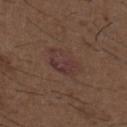Part of a total-body skin-imaging series; this lesion was reviewed on a skin check and was not flagged for biopsy. An algorithmic analysis of the crop reported a lesion color around L≈34 a*≈18 b*≈20 in CIELAB, roughly 5 lightness units darker than nearby skin, and a normalized border contrast of about 6. The tile uses white-light illumination. Measured at roughly 4 mm in maximum diameter. A male patient, aged approximately 50. The lesion is on the back. A close-up tile cropped from a whole-body skin photograph, about 15 mm across.